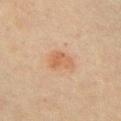Q: Was this lesion biopsied?
A: no biopsy performed (imaged during a skin exam)
Q: Who is the patient?
A: female, aged 43–47
Q: How was this image acquired?
A: 15 mm crop, total-body photography
Q: What is the anatomic site?
A: the chest
Q: How was the tile lit?
A: cross-polarized illumination
Q: What is the lesion's diameter?
A: ~3 mm (longest diameter)
Q: What did automated image analysis measure?
A: a footprint of about 5.5 mm², a shape eccentricity near 0.75, and two-axis asymmetry of about 0.35; a border-irregularity index near 3.5/10, internal color variation of about 2.5 on a 0–10 scale, and a peripheral color-asymmetry measure near 1; an automated nevus-likeness rating near 75 out of 100